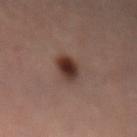The lesion is on the abdomen. A female patient, aged approximately 45. A 15 mm close-up tile from a total-body photography series done for melanoma screening. This is a cross-polarized tile.